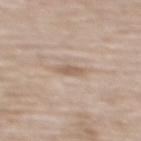workup = no biopsy performed (imaged during a skin exam)
image source = total-body-photography crop, ~15 mm field of view
site = the mid back
lighting = white-light
subject = female, aged around 75
size = ~3 mm (longest diameter)